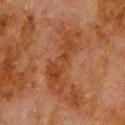Assessment: No biopsy was performed on this lesion — it was imaged during a full skin examination and was not determined to be concerning. Background: This is a cross-polarized tile. Cropped from a whole-body photographic skin survey; the tile spans about 15 mm. Automated image analysis of the tile measured a mean CIELAB color near L≈34 a*≈21 b*≈30 and a lesion-to-skin contrast of about 6 (normalized; higher = more distinct). The software also gave a classifier nevus-likeness of about 0/100 and lesion-presence confidence of about 100/100. Longest diameter approximately 7.5 mm. Located on the upper back. A male patient aged 78 to 82.